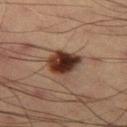follow-up: catalogued during a skin exam; not biopsied
imaging modality: total-body-photography crop, ~15 mm field of view
automated metrics: an average lesion color of about L≈28 a*≈18 b*≈23 (CIELAB) and a lesion–skin lightness drop of about 17; border irregularity of about 1.5 on a 0–10 scale, a within-lesion color-variation index near 6.5/10, and peripheral color asymmetry of about 2; a nevus-likeness score of about 100/100 and a lesion-detection confidence of about 100/100
subject: male, in their mid- to late 50s
diameter: ~4 mm (longest diameter)
site: the right thigh